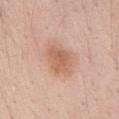An algorithmic analysis of the crop reported a footprint of about 11 mm² and a shape eccentricity near 0.65. The analysis additionally found a lesion–skin lightness drop of about 9 and a normalized lesion–skin contrast near 6.5. A lesion tile, about 15 mm wide, cut from a 3D total-body photograph. The subject is a male aged 53–57. The lesion is located on the abdomen. The lesion's longest dimension is about 4.5 mm.